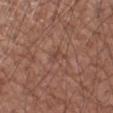Q: Is there a histopathology result?
A: no biopsy performed (imaged during a skin exam)
Q: How was this image acquired?
A: 15 mm crop, total-body photography
Q: What is the anatomic site?
A: the left upper arm
Q: How large is the lesion?
A: ~2.5 mm (longest diameter)
Q: What did automated image analysis measure?
A: border irregularity of about 4.5 on a 0–10 scale, internal color variation of about 1.5 on a 0–10 scale, and peripheral color asymmetry of about 0.5
Q: What are the patient's age and sex?
A: male, roughly 75 years of age